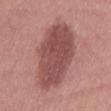workup: total-body-photography surveillance lesion; no biopsy | image source: 15 mm crop, total-body photography | size: about 12 mm | subject: female, approximately 40 years of age | lighting: white-light illumination | body site: the left thigh.